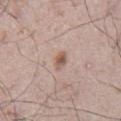follow-up = catalogued during a skin exam; not biopsied | image-analysis metrics = a mean CIELAB color near L≈57 a*≈18 b*≈25 and roughly 11 lightness units darker than nearby skin | body site = the front of the torso | size = ~2.5 mm (longest diameter) | image source = ~15 mm tile from a whole-body skin photo | subject = male, aged 63–67.